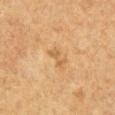Case summary:
• workup — total-body-photography surveillance lesion; no biopsy
• subject — male, approximately 65 years of age
• automated lesion analysis — a lesion color around L≈55 a*≈18 b*≈39 in CIELAB and roughly 7 lightness units darker than nearby skin
• diameter — ≈3 mm
• image — ~15 mm tile from a whole-body skin photo
• location — the chest
• illumination — cross-polarized illumination